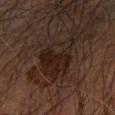Clinical impression:
The lesion was tiled from a total-body skin photograph and was not biopsied.
Image and clinical context:
Imaged with cross-polarized lighting. A male patient about 70 years old. Longest diameter approximately 7 mm. Located on the arm. Cropped from a whole-body photographic skin survey; the tile spans about 15 mm.